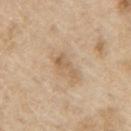Imaged during a routine full-body skin examination; the lesion was not biopsied and no histopathology is available. On the right upper arm. This is a white-light tile. The patient is a male in their 70s. About 3 mm across. Cropped from a whole-body photographic skin survey; the tile spans about 15 mm.